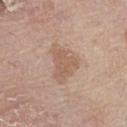Impression: The lesion was tiled from a total-body skin photograph and was not biopsied. Background: About 3.5 mm across. A 15 mm close-up tile from a total-body photography series done for melanoma screening. The lesion is on the leg. A male patient, roughly 75 years of age.